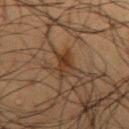This lesion was catalogued during total-body skin photography and was not selected for biopsy.
Imaged with cross-polarized lighting.
From the back.
A roughly 15 mm field-of-view crop from a total-body skin photograph.
A male patient, aged 58–62.